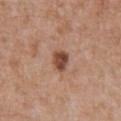No biopsy was performed on this lesion — it was imaged during a full skin examination and was not determined to be concerning.
From the upper back.
A male patient aged 58–62.
Imaged with white-light lighting.
Cropped from a whole-body photographic skin survey; the tile spans about 15 mm.